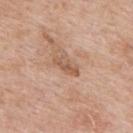follow-up = no biopsy performed (imaged during a skin exam) | diameter = ~3.5 mm (longest diameter) | body site = the upper back | lighting = white-light illumination | patient = male, aged 63–67 | image source = ~15 mm crop, total-body skin-cancer survey.